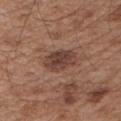{"biopsy_status": "not biopsied; imaged during a skin examination", "image": {"source": "total-body photography crop", "field_of_view_mm": 15}, "site": "left upper arm", "patient": {"sex": "male", "age_approx": 55}, "lighting": "white-light", "lesion_size": {"long_diameter_mm_approx": 4.0}}